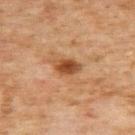Q: Automated lesion metrics?
A: an area of roughly 5 mm², an outline eccentricity of about 0.75 (0 = round, 1 = elongated), and a symmetry-axis asymmetry near 0.25; a border-irregularity rating of about 2.5/10, a within-lesion color-variation index near 4/10, and peripheral color asymmetry of about 1; an automated nevus-likeness rating near 95 out of 100
Q: How was the tile lit?
A: cross-polarized illumination
Q: How was this image acquired?
A: 15 mm crop, total-body photography
Q: Patient demographics?
A: female, aged around 60
Q: Where on the body is the lesion?
A: the upper back
Q: What is the lesion's diameter?
A: about 3 mm